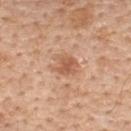Q: Was this lesion biopsied?
A: imaged on a skin check; not biopsied
Q: What kind of image is this?
A: total-body-photography crop, ~15 mm field of view
Q: What are the patient's age and sex?
A: male, about 60 years old
Q: How was the tile lit?
A: white-light illumination
Q: What is the lesion's diameter?
A: ~3 mm (longest diameter)
Q: What is the anatomic site?
A: the back